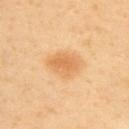biopsy_status: not biopsied; imaged during a skin examination
lesion_size:
  long_diameter_mm_approx: 4.0
lighting: cross-polarized
site: upper back
image:
  source: total-body photography crop
  field_of_view_mm: 15
patient:
  sex: female
  age_approx: 50